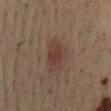Notes:
– notes: no biopsy performed (imaged during a skin exam)
– body site: the back
– automated metrics: an area of roughly 8.5 mm², an eccentricity of roughly 0.9, and a shape-asymmetry score of about 0.2 (0 = symmetric); an average lesion color of about L≈37 a*≈17 b*≈22 (CIELAB) and a lesion–skin lightness drop of about 8
– patient: male, approximately 55 years of age
– lighting: cross-polarized illumination
– lesion size: ≈5 mm
– imaging modality: total-body-photography crop, ~15 mm field of view